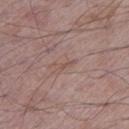Clinical impression:
The lesion was tiled from a total-body skin photograph and was not biopsied.
Clinical summary:
On the left lower leg. A lesion tile, about 15 mm wide, cut from a 3D total-body photograph. The patient is a male aged 63–67.